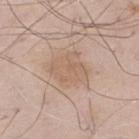follow-up: no biopsy performed (imaged during a skin exam); image-analysis metrics: a classifier nevus-likeness of about 0/100 and a detector confidence of about 100 out of 100 that the crop contains a lesion; diameter: ≈4.5 mm; patient: male, approximately 55 years of age; acquisition: ~15 mm tile from a whole-body skin photo; lighting: white-light; location: the left thigh.